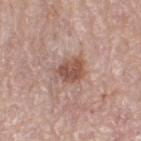Clinical summary:
Imaged with white-light lighting. A lesion tile, about 15 mm wide, cut from a 3D total-body photograph. Located on the right thigh. A female subject, aged 63–67.